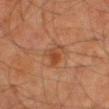Impression: The lesion was tiled from a total-body skin photograph and was not biopsied. Clinical summary: Cropped from a total-body skin-imaging series; the visible field is about 15 mm. A male subject approximately 80 years of age. On the leg.